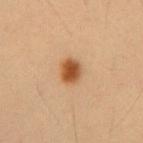Q: Is there a histopathology result?
A: catalogued during a skin exam; not biopsied
Q: Where on the body is the lesion?
A: the abdomen
Q: Patient demographics?
A: male, in their mid- to late 50s
Q: How large is the lesion?
A: ~3 mm (longest diameter)
Q: How was this image acquired?
A: ~15 mm crop, total-body skin-cancer survey
Q: Illumination type?
A: cross-polarized